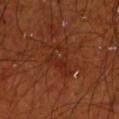Captured during whole-body skin photography for melanoma surveillance; the lesion was not biopsied. A close-up tile cropped from a whole-body skin photograph, about 15 mm across. From the left upper arm. A male subject approximately 70 years of age.